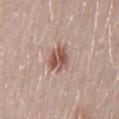Clinical impression: Part of a total-body skin-imaging series; this lesion was reviewed on a skin check and was not flagged for biopsy. Context: This is a white-light tile. The lesion is located on the back. Longest diameter approximately 3.5 mm. This image is a 15 mm lesion crop taken from a total-body photograph. The total-body-photography lesion software estimated a lesion area of about 7 mm² and a shape-asymmetry score of about 0.25 (0 = symmetric). And it measured a border-irregularity rating of about 2.5/10, a within-lesion color-variation index near 5.5/10, and peripheral color asymmetry of about 2. And it measured an automated nevus-likeness rating near 75 out of 100 and a lesion-detection confidence of about 100/100. A female subject, in their 30s.